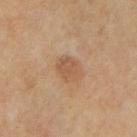Part of a total-body skin-imaging series; this lesion was reviewed on a skin check and was not flagged for biopsy. Cropped from a total-body skin-imaging series; the visible field is about 15 mm. The subject is a male aged approximately 65. The lesion is on the abdomen.